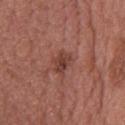A female subject aged 58 to 62. Approximately 2.5 mm at its widest. From the head or neck. A close-up tile cropped from a whole-body skin photograph, about 15 mm across. Captured under white-light illumination.